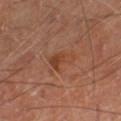Assessment:
This lesion was catalogued during total-body skin photography and was not selected for biopsy.
Image and clinical context:
From the right lower leg. The lesion-visualizer software estimated a lesion area of about 5.5 mm², a shape eccentricity near 0.7, and a symmetry-axis asymmetry near 0.4. It also reported an average lesion color of about L≈41 a*≈23 b*≈31 (CIELAB) and about 6 CIELAB-L* units darker than the surrounding skin. This image is a 15 mm lesion crop taken from a total-body photograph. Captured under cross-polarized illumination. About 3.5 mm across. A male subject, aged around 70.